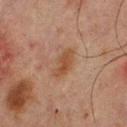notes: no biopsy performed (imaged during a skin exam); tile lighting: cross-polarized; size: ≈4 mm; imaging modality: ~15 mm tile from a whole-body skin photo; subject: male, about 65 years old; site: the upper back.